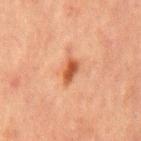{
  "biopsy_status": "not biopsied; imaged during a skin examination",
  "site": "abdomen",
  "patient": {
    "sex": "male",
    "age_approx": 65
  },
  "lighting": "cross-polarized",
  "image": {
    "source": "total-body photography crop",
    "field_of_view_mm": 15
  }
}